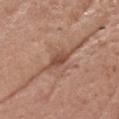No biopsy was performed on this lesion — it was imaged during a full skin examination and was not determined to be concerning. The lesion's longest dimension is about 3.5 mm. The subject is a male in their mid- to late 60s. A close-up tile cropped from a whole-body skin photograph, about 15 mm across. From the head or neck.